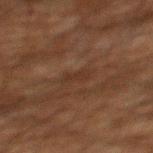The lesion was tiled from a total-body skin photograph and was not biopsied. A male subject aged 58 to 62. A region of skin cropped from a whole-body photographic capture, roughly 15 mm wide. The lesion is located on the mid back.